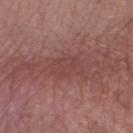{"biopsy_status": "not biopsied; imaged during a skin examination", "automated_metrics": {"area_mm2_approx": 8.5, "shape_asymmetry": 0.3, "vs_skin_darker_L": 6.0, "vs_skin_contrast_norm": 5.0, "border_irregularity_0_10": 3.5, "color_variation_0_10": 2.5, "peripheral_color_asymmetry": 1.0}, "image": {"source": "total-body photography crop", "field_of_view_mm": 15}, "patient": {"sex": "male", "age_approx": 55}, "site": "right forearm", "lesion_size": {"long_diameter_mm_approx": 4.5}}